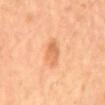Clinical impression: The lesion was photographed on a routine skin check and not biopsied; there is no pathology result.